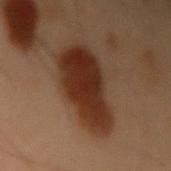Q: Is there a histopathology result?
A: catalogued during a skin exam; not biopsied
Q: Where on the body is the lesion?
A: the left upper arm
Q: Illumination type?
A: cross-polarized illumination
Q: Patient demographics?
A: male, aged 53–57
Q: How large is the lesion?
A: about 7.5 mm
Q: What is the imaging modality?
A: ~15 mm tile from a whole-body skin photo